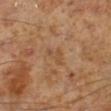<lesion>
  <site>left lower leg</site>
  <patient>
    <sex>male</sex>
    <age_approx>60</age_approx>
  </patient>
  <image>
    <source>total-body photography crop</source>
    <field_of_view_mm>15</field_of_view_mm>
  </image>
</lesion>